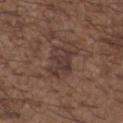Case summary:
* follow-up: total-body-photography surveillance lesion; no biopsy
* automated metrics: a lesion area of about 10 mm² and a shape-asymmetry score of about 0.25 (0 = symmetric); a mean CIELAB color near L≈36 a*≈16 b*≈20, a lesion–skin lightness drop of about 7, and a lesion-to-skin contrast of about 7.5 (normalized; higher = more distinct); border irregularity of about 4 on a 0–10 scale and a within-lesion color-variation index near 3.5/10
* lighting: white-light
* anatomic site: the chest
* size: ~4 mm (longest diameter)
* patient: male, about 50 years old
* image: ~15 mm tile from a whole-body skin photo